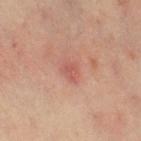Impression:
Imaged during a routine full-body skin examination; the lesion was not biopsied and no histopathology is available.
Clinical summary:
A 15 mm crop from a total-body photograph taken for skin-cancer surveillance. The lesion is on the right thigh. The total-body-photography lesion software estimated a nevus-likeness score of about 0/100 and lesion-presence confidence of about 100/100. The patient is a female aged 38 to 42.